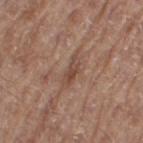This lesion was catalogued during total-body skin photography and was not selected for biopsy.
This is a white-light tile.
A male subject approximately 70 years of age.
A lesion tile, about 15 mm wide, cut from a 3D total-body photograph.
On the right thigh.